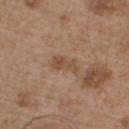Imaged during a routine full-body skin examination; the lesion was not biopsied and no histopathology is available. On the chest. Imaged with white-light lighting. Measured at roughly 3.5 mm in maximum diameter. A male patient aged around 55. A 15 mm close-up extracted from a 3D total-body photography capture. Automated tile analysis of the lesion measured a nevus-likeness score of about 0/100 and a lesion-detection confidence of about 100/100.